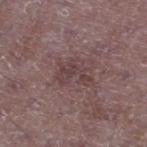Q: Was a biopsy performed?
A: total-body-photography surveillance lesion; no biopsy
Q: Lesion size?
A: ≈4 mm
Q: Who is the patient?
A: male, about 50 years old
Q: What is the imaging modality?
A: ~15 mm tile from a whole-body skin photo
Q: Illumination type?
A: white-light
Q: Where on the body is the lesion?
A: the left lower leg